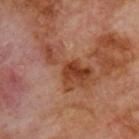Case summary:
- workup · imaged on a skin check; not biopsied
- automated lesion analysis · a lesion area of about 16 mm², a shape eccentricity near 0.9, and a symmetry-axis asymmetry near 0.55; an average lesion color of about L≈43 a*≈25 b*≈32 (CIELAB), roughly 10 lightness units darker than nearby skin, and a normalized border contrast of about 8.5; border irregularity of about 7.5 on a 0–10 scale, a within-lesion color-variation index near 6/10, and radial color variation of about 2
- size · about 7.5 mm
- acquisition · total-body-photography crop, ~15 mm field of view
- location · the upper back
- tile lighting · cross-polarized illumination
- patient · male, about 70 years old